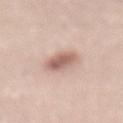Impression: No biopsy was performed on this lesion — it was imaged during a full skin examination and was not determined to be concerning. Context: On the lower back. Measured at roughly 3.5 mm in maximum diameter. Imaged with white-light lighting. The subject is a male aged 73 to 77. Cropped from a whole-body photographic skin survey; the tile spans about 15 mm.